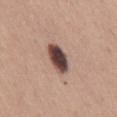No biopsy was performed on this lesion — it was imaged during a full skin examination and was not determined to be concerning.
The subject is a male aged approximately 65.
This is a white-light tile.
A lesion tile, about 15 mm wide, cut from a 3D total-body photograph.
The lesion is on the mid back.